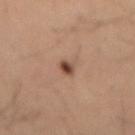Assessment: This lesion was catalogued during total-body skin photography and was not selected for biopsy. Context: Imaged with cross-polarized lighting. The lesion is on the mid back. The subject is a male aged 48 to 52. About 2 mm across. Cropped from a total-body skin-imaging series; the visible field is about 15 mm. The lesion-visualizer software estimated border irregularity of about 2 on a 0–10 scale and a peripheral color-asymmetry measure near 1.5.